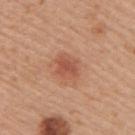biopsy_status: not biopsied; imaged during a skin examination